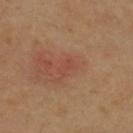{"biopsy_status": "not biopsied; imaged during a skin examination", "site": "upper back", "patient": {"sex": "female", "age_approx": 40}, "image": {"source": "total-body photography crop", "field_of_view_mm": 15}, "lesion_size": {"long_diameter_mm_approx": 2.0}}